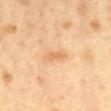<lesion>
  <biopsy_status>not biopsied; imaged during a skin examination</biopsy_status>
  <lighting>cross-polarized</lighting>
  <site>chest</site>
  <patient>
    <sex>female</sex>
    <age_approx>40</age_approx>
  </patient>
  <image>
    <source>total-body photography crop</source>
    <field_of_view_mm>15</field_of_view_mm>
  </image>
  <lesion_size>
    <long_diameter_mm_approx>2.5</long_diameter_mm_approx>
  </lesion_size>
</lesion>